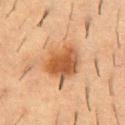Recorded during total-body skin imaging; not selected for excision or biopsy.
A male subject about 55 years old.
Automated tile analysis of the lesion measured a mean CIELAB color near L≈49 a*≈21 b*≈35, roughly 12 lightness units darker than nearby skin, and a lesion-to-skin contrast of about 9 (normalized; higher = more distinct). The software also gave a color-variation rating of about 6/10 and peripheral color asymmetry of about 2.
The lesion is on the upper back.
Measured at roughly 5 mm in maximum diameter.
A region of skin cropped from a whole-body photographic capture, roughly 15 mm wide.
Captured under cross-polarized illumination.